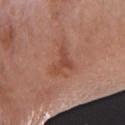Q: Is there a histopathology result?
A: catalogued during a skin exam; not biopsied
Q: What is the imaging modality?
A: 15 mm crop, total-body photography
Q: How large is the lesion?
A: ≈4 mm
Q: Who is the patient?
A: female, about 75 years old
Q: How was the tile lit?
A: white-light illumination
Q: Automated lesion metrics?
A: an area of roughly 6 mm² and a symmetry-axis asymmetry near 0.65; an average lesion color of about L≈48 a*≈25 b*≈30 (CIELAB), a lesion–skin lightness drop of about 8, and a normalized lesion–skin contrast near 6; a border-irregularity index near 7/10, a color-variation rating of about 2.5/10, and peripheral color asymmetry of about 0.5
Q: Lesion location?
A: the left forearm